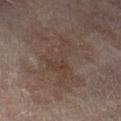Q: Was this lesion biopsied?
A: catalogued during a skin exam; not biopsied
Q: How large is the lesion?
A: ~7 mm (longest diameter)
Q: What is the imaging modality?
A: 15 mm crop, total-body photography
Q: Patient demographics?
A: female, aged 78 to 82
Q: Where on the body is the lesion?
A: the right leg
Q: What lighting was used for the tile?
A: cross-polarized